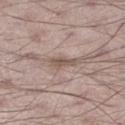- biopsy status: total-body-photography surveillance lesion; no biopsy
- imaging modality: total-body-photography crop, ~15 mm field of view
- patient: male, in their 70s
- lighting: white-light illumination
- location: the right thigh
- size: ~2.5 mm (longest diameter)